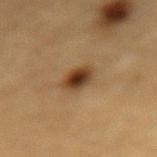The lesion was photographed on a routine skin check and not biopsied; there is no pathology result. An algorithmic analysis of the crop reported a border-irregularity rating of about 1.5/10, a color-variation rating of about 6.5/10, and peripheral color asymmetry of about 1.5. It also reported a classifier nevus-likeness of about 100/100 and lesion-presence confidence of about 100/100. From the mid back. Imaged with cross-polarized lighting. The patient is a male roughly 85 years of age or older. About 3 mm across. A 15 mm crop from a total-body photograph taken for skin-cancer surveillance.